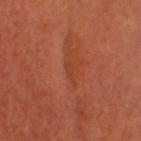Q: Who is the patient?
A: male, approximately 55 years of age
Q: Lesion location?
A: the head or neck
Q: How large is the lesion?
A: ~2 mm (longest diameter)
Q: What kind of image is this?
A: ~15 mm crop, total-body skin-cancer survey
Q: Illumination type?
A: cross-polarized